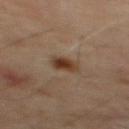Q: What is the imaging modality?
A: 15 mm crop, total-body photography
Q: What are the patient's age and sex?
A: male, roughly 65 years of age
Q: Where on the body is the lesion?
A: the mid back
Q: What lighting was used for the tile?
A: cross-polarized illumination
Q: Lesion size?
A: ~2.5 mm (longest diameter)
Q: Automated lesion metrics?
A: a shape eccentricity near 0.7 and two-axis asymmetry of about 0.2; a border-irregularity rating of about 2/10; a nevus-likeness score of about 95/100